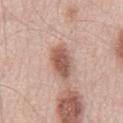  biopsy_status: not biopsied; imaged during a skin examination
  patient:
    sex: male
    age_approx: 55
  site: chest
  image:
    source: total-body photography crop
    field_of_view_mm: 15